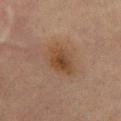biopsy_status: not biopsied; imaged during a skin examination
image:
  source: total-body photography crop
  field_of_view_mm: 15
patient:
  sex: male
  age_approx: 65
lighting: cross-polarized
site: mid back